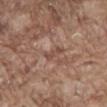{
  "biopsy_status": "not biopsied; imaged during a skin examination",
  "site": "abdomen",
  "lesion_size": {
    "long_diameter_mm_approx": 2.5
  },
  "lighting": "white-light",
  "patient": {
    "sex": "male",
    "age_approx": 80
  },
  "image": {
    "source": "total-body photography crop",
    "field_of_view_mm": 15
  }
}